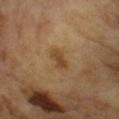Clinical impression:
Part of a total-body skin-imaging series; this lesion was reviewed on a skin check and was not flagged for biopsy.
Image and clinical context:
Cropped from a whole-body photographic skin survey; the tile spans about 15 mm. A male patient in their mid- to late 60s. Approximately 3 mm at its widest.